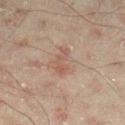Recorded during total-body skin imaging; not selected for excision or biopsy.
The tile uses cross-polarized illumination.
The lesion is on the right lower leg.
A lesion tile, about 15 mm wide, cut from a 3D total-body photograph.
A male patient aged 43 to 47.
The total-body-photography lesion software estimated a lesion color around L≈46 a*≈17 b*≈23 in CIELAB, a lesion–skin lightness drop of about 6, and a normalized lesion–skin contrast near 5.5.
Approximately 3.5 mm at its widest.